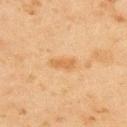{"biopsy_status": "not biopsied; imaged during a skin examination", "image": {"source": "total-body photography crop", "field_of_view_mm": 15}, "site": "upper back", "patient": {"sex": "male", "age_approx": 45}, "automated_metrics": {"cielab_L": 52, "cielab_a": 18, "cielab_b": 36, "vs_skin_darker_L": 7.0, "vs_skin_contrast_norm": 6.5}}